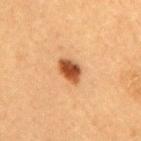notes = catalogued during a skin exam; not biopsied | subject = male, roughly 50 years of age | acquisition = ~15 mm crop, total-body skin-cancer survey | diameter = ~3 mm (longest diameter) | automated metrics = about 15 CIELAB-L* units darker than the surrounding skin and a normalized border contrast of about 11.5; a detector confidence of about 100 out of 100 that the crop contains a lesion | anatomic site = the upper back | illumination = cross-polarized.